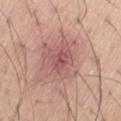Clinical impression: Part of a total-body skin-imaging series; this lesion was reviewed on a skin check and was not flagged for biopsy. Image and clinical context: A roughly 15 mm field-of-view crop from a total-body skin photograph. From the left thigh. Captured under white-light illumination. A male subject, aged around 60. Longest diameter approximately 2.5 mm.